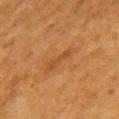{"biopsy_status": "not biopsied; imaged during a skin examination"}